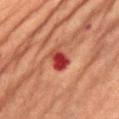{
  "biopsy_status": "not biopsied; imaged during a skin examination",
  "image": {
    "source": "total-body photography crop",
    "field_of_view_mm": 15
  },
  "patient": {
    "sex": "female",
    "age_approx": 70
  },
  "lighting": "cross-polarized",
  "lesion_size": {
    "long_diameter_mm_approx": 3.0
  },
  "site": "abdomen"
}